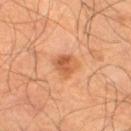Impression:
The lesion was tiled from a total-body skin photograph and was not biopsied.
Context:
Captured under cross-polarized illumination. A male patient, aged around 65. Automated image analysis of the tile measured a footprint of about 5.5 mm² and a shape eccentricity near 0.55. The software also gave a lesion color around L≈53 a*≈26 b*≈37 in CIELAB, roughly 10 lightness units darker than nearby skin, and a normalized border contrast of about 7. The analysis additionally found a border-irregularity index near 2.5/10, a color-variation rating of about 4.5/10, and radial color variation of about 1.5. A region of skin cropped from a whole-body photographic capture, roughly 15 mm wide. The lesion is on the left thigh.